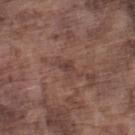follow-up = total-body-photography surveillance lesion; no biopsy | body site = the left lower leg | lesion diameter = ≈3 mm | acquisition = ~15 mm tile from a whole-body skin photo | illumination = white-light illumination | patient = male, in their mid-70s.